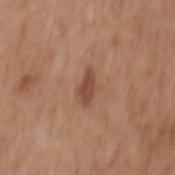{
  "biopsy_status": "not biopsied; imaged during a skin examination",
  "patient": {
    "sex": "male",
    "age_approx": 60
  },
  "image": {
    "source": "total-body photography crop",
    "field_of_view_mm": 15
  },
  "lesion_size": {
    "long_diameter_mm_approx": 3.5
  },
  "site": "mid back",
  "lighting": "white-light"
}